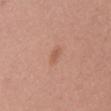biopsy status = total-body-photography surveillance lesion; no biopsy | lesion size = about 2.5 mm | illumination = white-light illumination | subject = female, aged 33–37 | acquisition = 15 mm crop, total-body photography | site = the mid back.